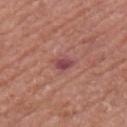This lesion was catalogued during total-body skin photography and was not selected for biopsy. The subject is a male approximately 80 years of age. This is a white-light tile. About 2.5 mm across. The lesion is on the left arm. The total-body-photography lesion software estimated an outline eccentricity of about 0.8 (0 = round, 1 = elongated) and a shape-asymmetry score of about 0.4 (0 = symmetric). The software also gave a within-lesion color-variation index near 2/10 and a peripheral color-asymmetry measure near 0.5. It also reported a nevus-likeness score of about 0/100 and lesion-presence confidence of about 100/100. A close-up tile cropped from a whole-body skin photograph, about 15 mm across.